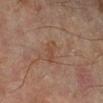workup: total-body-photography surveillance lesion; no biopsy
acquisition: 15 mm crop, total-body photography
diameter: ≈3 mm
site: the left lower leg
patient: male, aged approximately 65
lighting: cross-polarized